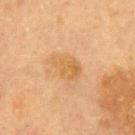Findings:
– subject · female, aged 58 to 62
– acquisition · total-body-photography crop, ~15 mm field of view
– lighting · cross-polarized
– lesion size · ~4 mm (longest diameter)
– image-analysis metrics · a lesion area of about 7 mm², a shape eccentricity near 0.8, and a shape-asymmetry score of about 0.25 (0 = symmetric); an automated nevus-likeness rating near 0 out of 100 and a lesion-detection confidence of about 100/100
– location · the mid back